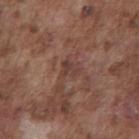workup: total-body-photography surveillance lesion; no biopsy
subject: male, approximately 75 years of age
image source: ~15 mm tile from a whole-body skin photo
lesion diameter: about 2.5 mm
automated lesion analysis: an area of roughly 4 mm², a shape eccentricity near 0.55, and a shape-asymmetry score of about 0.4 (0 = symmetric); an automated nevus-likeness rating near 0 out of 100 and a detector confidence of about 70 out of 100 that the crop contains a lesion
location: the chest
lighting: white-light